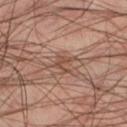biopsy status — catalogued during a skin exam; not biopsied | automated metrics — an area of roughly 3.5 mm² and an outline eccentricity of about 0.65 (0 = round, 1 = elongated); a mean CIELAB color near L≈45 a*≈18 b*≈26; border irregularity of about 7 on a 0–10 scale, a color-variation rating of about 0/10, and a peripheral color-asymmetry measure near 0 | subject — male, in their mid-40s | lighting — cross-polarized | body site — the left lower leg | imaging modality — ~15 mm tile from a whole-body skin photo.